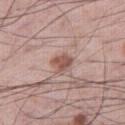• anatomic site — the leg
• subject — male, aged approximately 60
• automated metrics — an automated nevus-likeness rating near 90 out of 100
• image source — ~15 mm crop, total-body skin-cancer survey
• lesion size — about 2.5 mm